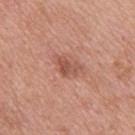{
  "biopsy_status": "not biopsied; imaged during a skin examination",
  "image": {
    "source": "total-body photography crop",
    "field_of_view_mm": 15
  },
  "patient": {
    "sex": "female",
    "age_approx": 45
  },
  "lesion_size": {
    "long_diameter_mm_approx": 3.0
  },
  "site": "upper back"
}